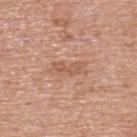A 15 mm crop from a total-body photograph taken for skin-cancer surveillance. The lesion is on the back. The lesion's longest dimension is about 3.5 mm. A female patient, aged 63 to 67.